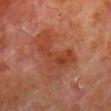| key | value |
|---|---|
| biopsy status | catalogued during a skin exam; not biopsied |
| illumination | cross-polarized |
| image source | 15 mm crop, total-body photography |
| location | the right lower leg |
| patient | male, in their 80s |
| lesion diameter | ~6 mm (longest diameter) |
| TBP lesion metrics | an eccentricity of roughly 0.9; a border-irregularity rating of about 7.5/10, a color-variation rating of about 3.5/10, and a peripheral color-asymmetry measure near 1; a nevus-likeness score of about 0/100 and a detector confidence of about 100 out of 100 that the crop contains a lesion |